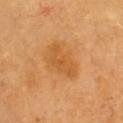tile lighting: cross-polarized; acquisition: ~15 mm crop, total-body skin-cancer survey; body site: the chest; subject: male, about 60 years old; lesion size: about 5 mm; automated metrics: an automated nevus-likeness rating near 60 out of 100.